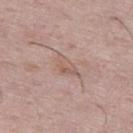Part of a total-body skin-imaging series; this lesion was reviewed on a skin check and was not flagged for biopsy.
The tile uses white-light illumination.
An algorithmic analysis of the crop reported a mean CIELAB color near L≈57 a*≈18 b*≈24, a lesion–skin lightness drop of about 7, and a normalized border contrast of about 5.
Longest diameter approximately 3 mm.
The lesion is located on the left thigh.
The patient is a male aged around 55.
A 15 mm close-up tile from a total-body photography series done for melanoma screening.